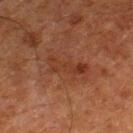| key | value |
|---|---|
| subject | male, aged around 80 |
| illumination | cross-polarized illumination |
| automated metrics | a footprint of about 7 mm², a shape eccentricity near 0.9, and two-axis asymmetry of about 0.4; a lesion-detection confidence of about 100/100 |
| lesion size | ≈4.5 mm |
| image source | 15 mm crop, total-body photography |
| location | the left thigh |